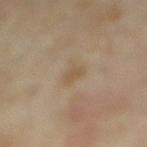<tbp_lesion>
<biopsy_status>not biopsied; imaged during a skin examination</biopsy_status>
<lighting>cross-polarized</lighting>
<patient>
  <sex>female</sex>
  <age_approx>35</age_approx>
</patient>
<image>
  <source>total-body photography crop</source>
  <field_of_view_mm>15</field_of_view_mm>
</image>
<site>right lower leg</site>
</tbp_lesion>